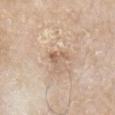Notes:
• notes: imaged on a skin check; not biopsied
• body site: the right upper arm
• image-analysis metrics: a footprint of about 3 mm² and an eccentricity of roughly 0.85; an average lesion color of about L≈61 a*≈17 b*≈31 (CIELAB) and a normalized border contrast of about 6.5
• imaging modality: ~15 mm tile from a whole-body skin photo
• subject: male, in their mid- to late 60s
• diameter: ≈2.5 mm
• tile lighting: white-light illumination